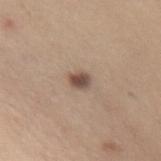Q: Was a biopsy performed?
A: catalogued during a skin exam; not biopsied
Q: Patient demographics?
A: female, aged around 40
Q: How was this image acquired?
A: 15 mm crop, total-body photography
Q: Lesion size?
A: about 2.5 mm
Q: Illumination type?
A: white-light illumination
Q: Lesion location?
A: the abdomen